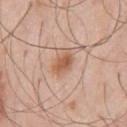Impression: Recorded during total-body skin imaging; not selected for excision or biopsy. Acquisition and patient details: The lesion is located on the chest. A 15 mm crop from a total-body photograph taken for skin-cancer surveillance. The tile uses white-light illumination. Approximately 3.5 mm at its widest. Automated image analysis of the tile measured a lesion color around L≈58 a*≈21 b*≈33 in CIELAB, a lesion–skin lightness drop of about 10, and a normalized border contrast of about 7.5. It also reported a nevus-likeness score of about 95/100. The subject is a male approximately 55 years of age.